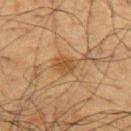<lesion>
<biopsy_status>not biopsied; imaged during a skin examination</biopsy_status>
<lighting>cross-polarized</lighting>
<automated_metrics>
  <area_mm2_approx>5.0</area_mm2_approx>
  <eccentricity>0.75</eccentricity>
  <shape_asymmetry>0.3</shape_asymmetry>
  <border_irregularity_0_10>3.0</border_irregularity_0_10>
  <color_variation_0_10>2.0</color_variation_0_10>
  <peripheral_color_asymmetry>1.0</peripheral_color_asymmetry>
</automated_metrics>
<image>
  <source>total-body photography crop</source>
  <field_of_view_mm>15</field_of_view_mm>
</image>
<patient>
  <sex>male</sex>
  <age_approx>60</age_approx>
</patient>
<site>upper back</site>
<lesion_size>
  <long_diameter_mm_approx>3.5</long_diameter_mm_approx>
</lesion_size>
</lesion>